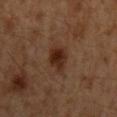* workup — catalogued during a skin exam; not biopsied
* image-analysis metrics — an outline eccentricity of about 0.8 (0 = round, 1 = elongated) and two-axis asymmetry of about 0.25; an automated nevus-likeness rating near 95 out of 100 and lesion-presence confidence of about 100/100
* patient — male, roughly 60 years of age
* image source — ~15 mm crop, total-body skin-cancer survey
* size — ~4 mm (longest diameter)
* lighting — cross-polarized
* site — the mid back